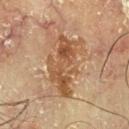Recorded during total-body skin imaging; not selected for excision or biopsy. Located on the chest. The tile uses cross-polarized illumination. A region of skin cropped from a whole-body photographic capture, roughly 15 mm wide. The lesion's longest dimension is about 7 mm. A male subject aged around 70.